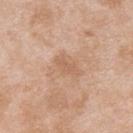The lesion was tiled from a total-body skin photograph and was not biopsied.
A male subject, about 25 years old.
Longest diameter approximately 3 mm.
Captured under white-light illumination.
Located on the back.
A roughly 15 mm field-of-view crop from a total-body skin photograph.